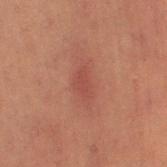{
  "biopsy_status": "not biopsied; imaged during a skin examination",
  "image": {
    "source": "total-body photography crop",
    "field_of_view_mm": 15
  },
  "patient": {
    "sex": "female",
    "age_approx": 80
  },
  "site": "right thigh"
}